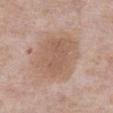Captured under white-light illumination.
A lesion tile, about 15 mm wide, cut from a 3D total-body photograph.
A male patient about 75 years old.
The lesion is on the chest.